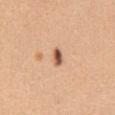{
  "biopsy_status": "not biopsied; imaged during a skin examination",
  "patient": {
    "sex": "female",
    "age_approx": 45
  },
  "site": "abdomen",
  "image": {
    "source": "total-body photography crop",
    "field_of_view_mm": 15
  }
}